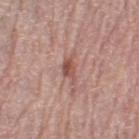Impression:
The lesion was tiled from a total-body skin photograph and was not biopsied.
Image and clinical context:
Automated tile analysis of the lesion measured an average lesion color of about L≈52 a*≈23 b*≈25 (CIELAB), roughly 11 lightness units darker than nearby skin, and a lesion-to-skin contrast of about 7.5 (normalized; higher = more distinct). The analysis additionally found border irregularity of about 4.5 on a 0–10 scale, a within-lesion color-variation index near 3/10, and peripheral color asymmetry of about 1. And it measured a classifier nevus-likeness of about 0/100. Approximately 3.5 mm at its widest. The subject is a female in their mid- to late 60s. Cropped from a total-body skin-imaging series; the visible field is about 15 mm. Imaged with white-light lighting. The lesion is located on the left thigh.